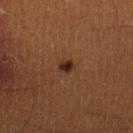follow-up — total-body-photography surveillance lesion; no biopsy
tile lighting — cross-polarized
lesion size — ~2 mm (longest diameter)
body site — the right lower leg
patient — male, aged approximately 40
image — ~15 mm crop, total-body skin-cancer survey